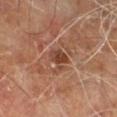follow-up — total-body-photography surveillance lesion; no biopsy
patient — male, roughly 60 years of age
size — ~3 mm (longest diameter)
tile lighting — cross-polarized illumination
TBP lesion metrics — a footprint of about 4.5 mm², an outline eccentricity of about 0.7 (0 = round, 1 = elongated), and a symmetry-axis asymmetry near 0.35; a border-irregularity rating of about 4/10, a color-variation rating of about 3/10, and radial color variation of about 1
anatomic site — the right leg
acquisition — 15 mm crop, total-body photography